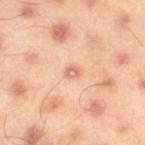Q: Was this lesion biopsied?
A: catalogued during a skin exam; not biopsied
Q: What are the patient's age and sex?
A: male, aged 43–47
Q: What kind of image is this?
A: ~15 mm crop, total-body skin-cancer survey
Q: Where on the body is the lesion?
A: the right thigh